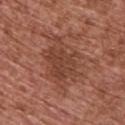The lesion was photographed on a routine skin check and not biopsied; there is no pathology result. A male patient, aged 73–77. On the back. Cropped from a total-body skin-imaging series; the visible field is about 15 mm. About 7.5 mm across.